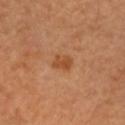No biopsy was performed on this lesion — it was imaged during a full skin examination and was not determined to be concerning.
The patient is a male aged approximately 60.
A roughly 15 mm field-of-view crop from a total-body skin photograph.
From the head or neck.
Imaged with cross-polarized lighting.
The lesion's longest dimension is about 2.5 mm.